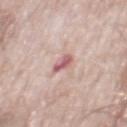workup: catalogued during a skin exam; not biopsied | acquisition: ~15 mm crop, total-body skin-cancer survey | subject: male, aged approximately 75 | anatomic site: the mid back.